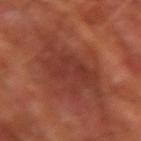notes = no biopsy performed (imaged during a skin exam)
acquisition = ~15 mm tile from a whole-body skin photo
size = about 6.5 mm
illumination = cross-polarized
patient = male, aged 63 to 67
site = the right upper arm